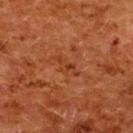Impression: The lesion was tiled from a total-body skin photograph and was not biopsied. Image and clinical context: From the upper back. Cropped from a total-body skin-imaging series; the visible field is about 15 mm. A female subject, aged approximately 50.